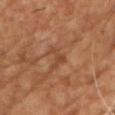{
  "biopsy_status": "not biopsied; imaged during a skin examination",
  "lighting": "cross-polarized",
  "site": "chest",
  "image": {
    "source": "total-body photography crop",
    "field_of_view_mm": 15
  },
  "patient": {
    "sex": "male",
    "age_approx": 55
  }
}